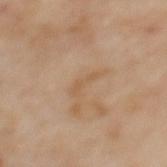No biopsy was performed on this lesion — it was imaged during a full skin examination and was not determined to be concerning. A female patient, aged around 60. Cropped from a total-body skin-imaging series; the visible field is about 15 mm. Captured under cross-polarized illumination. The lesion is on the upper back. The total-body-photography lesion software estimated roughly 5 lightness units darker than nearby skin and a normalized lesion–skin contrast near 4.5. The analysis additionally found a nevus-likeness score of about 0/100. Longest diameter approximately 2.5 mm.